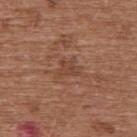Clinical impression: No biopsy was performed on this lesion — it was imaged during a full skin examination and was not determined to be concerning. Background: The lesion's longest dimension is about 3 mm. A lesion tile, about 15 mm wide, cut from a 3D total-body photograph. The lesion-visualizer software estimated a lesion area of about 4 mm², an eccentricity of roughly 0.65, and a symmetry-axis asymmetry near 0.45. The analysis additionally found a mean CIELAB color near L≈44 a*≈22 b*≈29, roughly 7 lightness units darker than nearby skin, and a lesion-to-skin contrast of about 5.5 (normalized; higher = more distinct). It also reported a border-irregularity rating of about 5/10, internal color variation of about 2 on a 0–10 scale, and radial color variation of about 1. On the upper back. The tile uses white-light illumination. A male patient, aged 73–77.